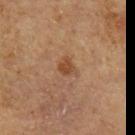The lesion was tiled from a total-body skin photograph and was not biopsied. Cropped from a whole-body photographic skin survey; the tile spans about 15 mm. Imaged with cross-polarized lighting. From the left upper arm. The lesion-visualizer software estimated a detector confidence of about 100 out of 100 that the crop contains a lesion. The subject is a male in their mid- to late 60s. Approximately 2.5 mm at its widest.